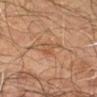Q: Was a biopsy performed?
A: no biopsy performed (imaged during a skin exam)
Q: What is the anatomic site?
A: the left forearm
Q: How was this image acquired?
A: ~15 mm tile from a whole-body skin photo
Q: What are the patient's age and sex?
A: male, aged 63 to 67
Q: What lighting was used for the tile?
A: cross-polarized illumination